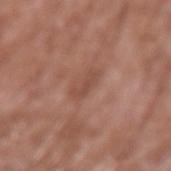Assessment: This lesion was catalogued during total-body skin photography and was not selected for biopsy. Context: A male patient, aged 58 to 62. Located on the left upper arm. The lesion-visualizer software estimated a footprint of about 2.5 mm² and a symmetry-axis asymmetry near 0.35. It also reported internal color variation of about 0 on a 0–10 scale and peripheral color asymmetry of about 0. It also reported an automated nevus-likeness rating near 0 out of 100 and lesion-presence confidence of about 100/100. Longest diameter approximately 2.5 mm. A 15 mm close-up extracted from a 3D total-body photography capture. Captured under white-light illumination.